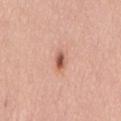Findings:
– workup: total-body-photography surveillance lesion; no biopsy
– subject: male, in their mid- to late 50s
– automated lesion analysis: a lesion color around L≈58 a*≈25 b*≈30 in CIELAB and a normalized lesion–skin contrast near 9
– imaging modality: ~15 mm crop, total-body skin-cancer survey
– location: the mid back
– diameter: ≈2.5 mm
– illumination: white-light